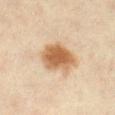Part of a total-body skin-imaging series; this lesion was reviewed on a skin check and was not flagged for biopsy. The tile uses cross-polarized illumination. A female subject in their mid- to late 40s. Cropped from a total-body skin-imaging series; the visible field is about 15 mm. An algorithmic analysis of the crop reported a mean CIELAB color near L≈52 a*≈18 b*≈33, about 13 CIELAB-L* units darker than the surrounding skin, and a normalized lesion–skin contrast near 10. And it measured border irregularity of about 2 on a 0–10 scale and peripheral color asymmetry of about 1. And it measured a nevus-likeness score of about 100/100 and lesion-presence confidence of about 100/100. On the right lower leg.